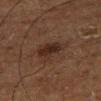{"biopsy_status": "not biopsied; imaged during a skin examination", "lighting": "cross-polarized", "patient": {"sex": "male", "age_approx": 75}, "site": "right thigh", "image": {"source": "total-body photography crop", "field_of_view_mm": 15}}